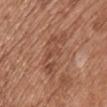| key | value |
|---|---|
| biopsy status | catalogued during a skin exam; not biopsied |
| image source | ~15 mm tile from a whole-body skin photo |
| automated lesion analysis | two-axis asymmetry of about 0.35; a classifier nevus-likeness of about 0/100 and a lesion-detection confidence of about 100/100 |
| subject | male, about 65 years old |
| tile lighting | white-light illumination |
| anatomic site | the front of the torso |